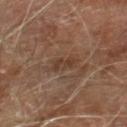Assessment: The lesion was tiled from a total-body skin photograph and was not biopsied. Clinical summary: A roughly 15 mm field-of-view crop from a total-body skin photograph. Imaged with cross-polarized lighting. A male subject aged approximately 70. The lesion is on the leg.